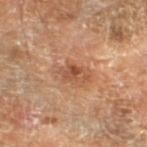Impression: Imaged during a routine full-body skin examination; the lesion was not biopsied and no histopathology is available. Context: A male patient aged around 70. Imaged with cross-polarized lighting. Cropped from a total-body skin-imaging series; the visible field is about 15 mm. About 3 mm across. Located on the left lower leg. The lesion-visualizer software estimated a footprint of about 4 mm², an outline eccentricity of about 0.8 (0 = round, 1 = elongated), and a symmetry-axis asymmetry near 0.25. The software also gave a lesion color around L≈50 a*≈24 b*≈33 in CIELAB, about 10 CIELAB-L* units darker than the surrounding skin, and a normalized border contrast of about 7. The analysis additionally found a classifier nevus-likeness of about 15/100 and a detector confidence of about 100 out of 100 that the crop contains a lesion.